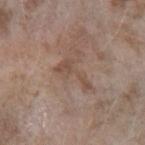notes = catalogued during a skin exam; not biopsied
image source = ~15 mm tile from a whole-body skin photo
anatomic site = the left forearm
patient = female, aged approximately 75